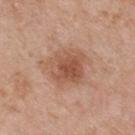Captured during whole-body skin photography for melanoma surveillance; the lesion was not biopsied. Imaged with white-light lighting. A lesion tile, about 15 mm wide, cut from a 3D total-body photograph. On the upper back. The patient is a male in their mid- to late 50s.